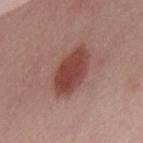No biopsy was performed on this lesion — it was imaged during a full skin examination and was not determined to be concerning.
The tile uses white-light illumination.
A male subject in their 40s.
About 6 mm across.
A region of skin cropped from a whole-body photographic capture, roughly 15 mm wide.
Located on the upper back.